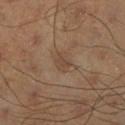{
  "biopsy_status": "not biopsied; imaged during a skin examination",
  "lighting": "cross-polarized",
  "patient": {
    "sex": "male",
    "age_approx": 45
  },
  "site": "right lower leg",
  "lesion_size": {
    "long_diameter_mm_approx": 2.5
  },
  "image": {
    "source": "total-body photography crop",
    "field_of_view_mm": 15
  },
  "automated_metrics": {
    "border_irregularity_0_10": 2.0,
    "color_variation_0_10": 1.5,
    "peripheral_color_asymmetry": 0.5,
    "nevus_likeness_0_100": 0
  }
}